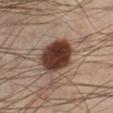biopsy status: no biopsy performed (imaged during a skin exam) | automated metrics: an area of roughly 18 mm², an outline eccentricity of about 0.7 (0 = round, 1 = elongated), and two-axis asymmetry of about 0.15; a nevus-likeness score of about 100/100 and lesion-presence confidence of about 100/100 | image: total-body-photography crop, ~15 mm field of view | anatomic site: the left lower leg | lighting: cross-polarized illumination | subject: male, roughly 40 years of age.